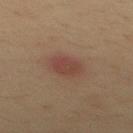Q: Was a biopsy performed?
A: catalogued during a skin exam; not biopsied
Q: Who is the patient?
A: male, in their mid- to late 30s
Q: What kind of image is this?
A: ~15 mm tile from a whole-body skin photo
Q: Lesion size?
A: ≈3 mm
Q: Automated lesion metrics?
A: an area of roughly 6 mm², an eccentricity of roughly 0.65, and a symmetry-axis asymmetry near 0.2
Q: Where on the body is the lesion?
A: the back